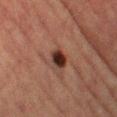Part of a total-body skin-imaging series; this lesion was reviewed on a skin check and was not flagged for biopsy. Captured under cross-polarized illumination. Cropped from a total-body skin-imaging series; the visible field is about 15 mm. The lesion is located on the left thigh. The lesion's longest dimension is about 2.5 mm. The subject is a female aged around 55.